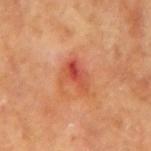Clinical impression:
Recorded during total-body skin imaging; not selected for excision or biopsy.
Background:
A female subject, aged 63 to 67. Located on the left forearm. Measured at roughly 4 mm in maximum diameter. Captured under cross-polarized illumination. A 15 mm close-up tile from a total-body photography series done for melanoma screening.